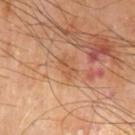notes: no biopsy performed (imaged during a skin exam) | automated lesion analysis: an area of roughly 2.5 mm²; a mean CIELAB color near L≈55 a*≈23 b*≈37 and a lesion–skin lightness drop of about 7 | patient: male, aged 63–67 | site: the arm | tile lighting: cross-polarized illumination | image source: total-body-photography crop, ~15 mm field of view.